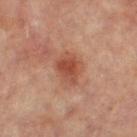  biopsy_status: not biopsied; imaged during a skin examination
  lighting: cross-polarized
  site: left leg
  image:
    source: total-body photography crop
    field_of_view_mm: 15
  automated_metrics:
    area_mm2_approx: 8.0
    eccentricity: 0.7
    shape_asymmetry: 0.2
    nevus_likeness_0_100: 90
  patient:
    sex: female
    age_approx: 65
  lesion_size:
    long_diameter_mm_approx: 4.0